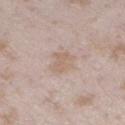follow-up=total-body-photography surveillance lesion; no biopsy | TBP lesion metrics=a footprint of about 6.5 mm², an eccentricity of roughly 0.35, and a symmetry-axis asymmetry near 0.3; a normalized border contrast of about 5 | location=the left lower leg | image source=~15 mm crop, total-body skin-cancer survey | subject=female, aged approximately 25 | lighting=white-light illumination | lesion size=≈3 mm.